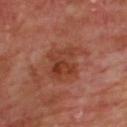This lesion was catalogued during total-body skin photography and was not selected for biopsy.
A region of skin cropped from a whole-body photographic capture, roughly 15 mm wide.
Approximately 5.5 mm at its widest.
From the upper back.
Captured under cross-polarized illumination.
Automated tile analysis of the lesion measured an area of roughly 14 mm², an outline eccentricity of about 0.65 (0 = round, 1 = elongated), and two-axis asymmetry of about 0.3. The analysis additionally found a lesion–skin lightness drop of about 7 and a normalized border contrast of about 7. The analysis additionally found a lesion-detection confidence of about 100/100.
A male subject, aged around 65.